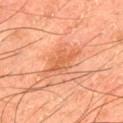Clinical impression: Imaged during a routine full-body skin examination; the lesion was not biopsied and no histopathology is available. Acquisition and patient details: Cropped from a whole-body photographic skin survey; the tile spans about 15 mm. A male subject aged 43–47. The lesion-visualizer software estimated an area of roughly 8 mm², an outline eccentricity of about 0.8 (0 = round, 1 = elongated), and a symmetry-axis asymmetry near 0.4. The analysis additionally found an average lesion color of about L≈59 a*≈29 b*≈39 (CIELAB), about 8 CIELAB-L* units darker than the surrounding skin, and a normalized lesion–skin contrast near 6. And it measured a color-variation rating of about 2.5/10 and peripheral color asymmetry of about 1. The software also gave an automated nevus-likeness rating near 0 out of 100 and a lesion-detection confidence of about 100/100. Captured under cross-polarized illumination. The lesion is located on the back. Measured at roughly 4.5 mm in maximum diameter.